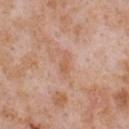No biopsy was performed on this lesion — it was imaged during a full skin examination and was not determined to be concerning.
A male patient aged around 65.
A close-up tile cropped from a whole-body skin photograph, about 15 mm across.
On the chest.